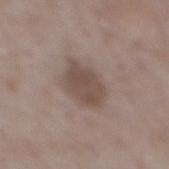Imaged during a routine full-body skin examination; the lesion was not biopsied and no histopathology is available.
About 4.5 mm across.
The tile uses white-light illumination.
A female subject, aged approximately 70.
Cropped from a whole-body photographic skin survey; the tile spans about 15 mm.
From the upper back.